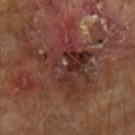  automated_metrics:
    area_mm2_approx: 25.0
    eccentricity: 0.7
    shape_asymmetry: 0.4
    cielab_L: 30
    cielab_a: 20
    cielab_b: 22
    vs_skin_contrast_norm: 7.5
  image:
    source: total-body photography crop
    field_of_view_mm: 15
  lesion_size:
    long_diameter_mm_approx: 8.5
  site: left forearm
  patient:
    sex: male
    age_approx: 65
  lighting: cross-polarized